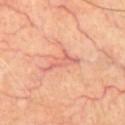workup=catalogued during a skin exam; not biopsied
patient=male, about 60 years old
anatomic site=the chest
lighting=cross-polarized illumination
imaging modality=15 mm crop, total-body photography